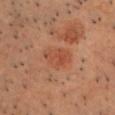| field | value |
|---|---|
| follow-up | total-body-photography surveillance lesion; no biopsy |
| anatomic site | the chest |
| automated lesion analysis | a footprint of about 8 mm² and two-axis asymmetry of about 0.3; a mean CIELAB color near L≈49 a*≈27 b*≈33 and a normalized border contrast of about 5; a nevus-likeness score of about 35/100 and lesion-presence confidence of about 100/100 |
| image source | 15 mm crop, total-body photography |
| illumination | cross-polarized |
| size | ~4 mm (longest diameter) |
| patient | male, approximately 65 years of age |